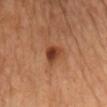Cropped from a whole-body photographic skin survey; the tile spans about 15 mm.
This is a cross-polarized tile.
A male subject, in their 70s.
On the chest.
The total-body-photography lesion software estimated a lesion area of about 5 mm² and an outline eccentricity of about 0.55 (0 = round, 1 = elongated). It also reported border irregularity of about 2.5 on a 0–10 scale, a within-lesion color-variation index near 5.5/10, and a peripheral color-asymmetry measure near 2. And it measured a classifier nevus-likeness of about 75/100 and lesion-presence confidence of about 100/100.